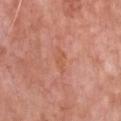Case summary:
– workup · imaged on a skin check; not biopsied
– subject · male, approximately 80 years of age
– anatomic site · the chest
– lighting · white-light illumination
– lesion diameter · ≈3 mm
– acquisition · total-body-photography crop, ~15 mm field of view